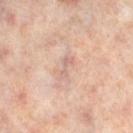workup = imaged on a skin check; not biopsied | size = ≈2.5 mm | tile lighting = cross-polarized | subject = female, about 50 years old | acquisition = ~15 mm tile from a whole-body skin photo | site = the right lower leg | image-analysis metrics = a border-irregularity index near 5/10 and internal color variation of about 0 on a 0–10 scale; a classifier nevus-likeness of about 0/100 and lesion-presence confidence of about 85/100.